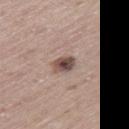Part of a total-body skin-imaging series; this lesion was reviewed on a skin check and was not flagged for biopsy.
From the right thigh.
A male patient about 65 years old.
A region of skin cropped from a whole-body photographic capture, roughly 15 mm wide.
The tile uses white-light illumination.